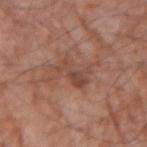| field | value |
|---|---|
| notes | catalogued during a skin exam; not biopsied |
| anatomic site | the right upper arm |
| patient | male, in their mid-70s |
| acquisition | ~15 mm tile from a whole-body skin photo |
| lighting | white-light illumination |